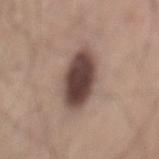biopsy_status: not biopsied; imaged during a skin examination
automated_metrics:
  eccentricity: 0.8
  shape_asymmetry: 0.1
  nevus_likeness_0_100: 90
  lesion_detection_confidence_0_100: 100
site: back
image:
  source: total-body photography crop
  field_of_view_mm: 15
patient:
  sex: male
  age_approx: 60